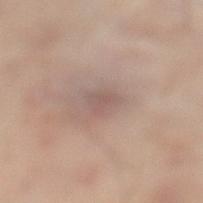follow-up = imaged on a skin check; not biopsied | acquisition = ~15 mm crop, total-body skin-cancer survey | patient = male, in their 50s | body site = the left lower leg.